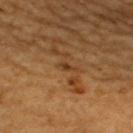The lesion was tiled from a total-body skin photograph and was not biopsied.
A region of skin cropped from a whole-body photographic capture, roughly 15 mm wide.
A male patient, roughly 60 years of age.
Located on the upper back.